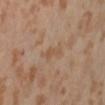Context: This is a cross-polarized tile. On the abdomen. A roughly 15 mm field-of-view crop from a total-body skin photograph. The patient is a female in their mid- to late 50s.